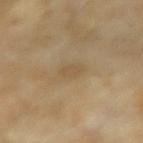Findings:
– biopsy status: total-body-photography surveillance lesion; no biopsy
– image: total-body-photography crop, ~15 mm field of view
– anatomic site: the left forearm
– image-analysis metrics: an area of roughly 3.5 mm² and an eccentricity of roughly 0.75; a border-irregularity index near 2.5/10 and radial color variation of about 0.5
– lesion size: about 2.5 mm
– patient: female, aged 73 to 77